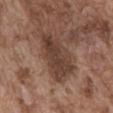This lesion was catalogued during total-body skin photography and was not selected for biopsy. The total-body-photography lesion software estimated an eccentricity of roughly 0.85 and a symmetry-axis asymmetry near 0.25. The analysis additionally found an automated nevus-likeness rating near 5 out of 100. The lesion is located on the front of the torso. This is a white-light tile. A lesion tile, about 15 mm wide, cut from a 3D total-body photograph. A male patient, aged 73–77.